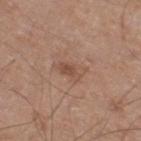subject: male, approximately 60 years of age
image source: ~15 mm tile from a whole-body skin photo
diameter: about 4 mm
anatomic site: the leg